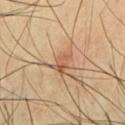{
  "biopsy_status": "not biopsied; imaged during a skin examination",
  "automated_metrics": {
    "area_mm2_approx": 4.5,
    "eccentricity": 0.75,
    "shape_asymmetry": 0.35,
    "border_irregularity_0_10": 3.5,
    "peripheral_color_asymmetry": 2.0,
    "nevus_likeness_0_100": 5,
    "lesion_detection_confidence_0_100": 100
  },
  "lesion_size": {
    "long_diameter_mm_approx": 3.0
  },
  "lighting": "cross-polarized",
  "patient": {
    "sex": "male",
    "age_approx": 40
  },
  "site": "chest",
  "image": {
    "source": "total-body photography crop",
    "field_of_view_mm": 15
  }
}